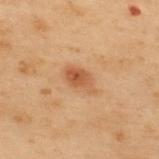Recorded during total-body skin imaging; not selected for excision or biopsy.
Imaged with cross-polarized lighting.
A male subject aged 53 to 57.
An algorithmic analysis of the crop reported a footprint of about 6 mm², an outline eccentricity of about 0.85 (0 = round, 1 = elongated), and a symmetry-axis asymmetry near 0.35. It also reported an average lesion color of about L≈45 a*≈20 b*≈32 (CIELAB) and roughly 9 lightness units darker than nearby skin. The analysis additionally found a border-irregularity rating of about 3.5/10, a color-variation rating of about 4/10, and peripheral color asymmetry of about 1.5. The software also gave an automated nevus-likeness rating near 65 out of 100 and a lesion-detection confidence of about 100/100.
A lesion tile, about 15 mm wide, cut from a 3D total-body photograph.
The lesion's longest dimension is about 3.5 mm.
On the upper back.